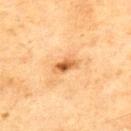Impression: The lesion was tiled from a total-body skin photograph and was not biopsied. Context: A 15 mm crop from a total-body photograph taken for skin-cancer surveillance. The lesion is located on the upper back. The total-body-photography lesion software estimated an average lesion color of about L≈51 a*≈22 b*≈39 (CIELAB), about 13 CIELAB-L* units darker than the surrounding skin, and a normalized border contrast of about 9. The recorded lesion diameter is about 3 mm. This is a cross-polarized tile. A male patient aged approximately 70.